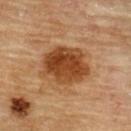biopsy status: imaged on a skin check; not biopsied
subject: male, approximately 85 years of age or older
site: the upper back
imaging modality: ~15 mm tile from a whole-body skin photo